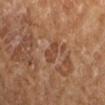Clinical impression:
Captured during whole-body skin photography for melanoma surveillance; the lesion was not biopsied.
Acquisition and patient details:
A 15 mm close-up extracted from a 3D total-body photography capture. About 3 mm across. The tile uses cross-polarized illumination. A female subject, about 70 years old. From the arm.